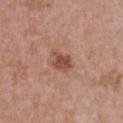Longest diameter approximately 2.5 mm. Captured under white-light illumination. Cropped from a total-body skin-imaging series; the visible field is about 15 mm. Located on the chest. A female patient, roughly 30 years of age. The total-body-photography lesion software estimated a shape eccentricity near 0.65.